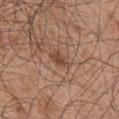The lesion was photographed on a routine skin check and not biopsied; there is no pathology result. A 15 mm close-up tile from a total-body photography series done for melanoma screening. Captured under white-light illumination. The patient is a male in their mid-40s. On the right upper arm. Approximately 3 mm at its widest.